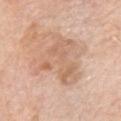Assessment: Recorded during total-body skin imaging; not selected for excision or biopsy. Background: A 15 mm close-up tile from a total-body photography series done for melanoma screening. From the chest. The lesion's longest dimension is about 6.5 mm. Imaged with white-light lighting. A male subject, about 80 years old.